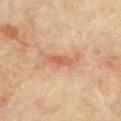This lesion was catalogued during total-body skin photography and was not selected for biopsy.
The lesion's longest dimension is about 5 mm.
The tile uses cross-polarized illumination.
On the chest.
An algorithmic analysis of the crop reported a shape-asymmetry score of about 0.35 (0 = symmetric). The analysis additionally found an average lesion color of about L≈59 a*≈24 b*≈33 (CIELAB), a lesion–skin lightness drop of about 9, and a normalized border contrast of about 6. And it measured an automated nevus-likeness rating near 0 out of 100 and a detector confidence of about 100 out of 100 that the crop contains a lesion.
The subject is a male aged 63–67.
This image is a 15 mm lesion crop taken from a total-body photograph.